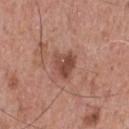The lesion was photographed on a routine skin check and not biopsied; there is no pathology result.